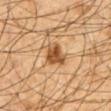Assessment:
Recorded during total-body skin imaging; not selected for excision or biopsy.
Image and clinical context:
On the chest. Captured under cross-polarized illumination. Automated tile analysis of the lesion measured two-axis asymmetry of about 0.3. The software also gave a lesion–skin lightness drop of about 12 and a normalized border contrast of about 9.5. It also reported border irregularity of about 3 on a 0–10 scale, internal color variation of about 4.5 on a 0–10 scale, and radial color variation of about 1.5. It also reported an automated nevus-likeness rating near 90 out of 100 and a detector confidence of about 100 out of 100 that the crop contains a lesion. Approximately 3.5 mm at its widest. A male patient, in their mid-60s. A roughly 15 mm field-of-view crop from a total-body skin photograph.